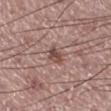Notes:
– follow-up — total-body-photography surveillance lesion; no biopsy
– automated metrics — an area of roughly 4 mm², an outline eccentricity of about 0.7 (0 = round, 1 = elongated), and two-axis asymmetry of about 0.2; a border-irregularity rating of about 2.5/10, internal color variation of about 4 on a 0–10 scale, and peripheral color asymmetry of about 1.5; a nevus-likeness score of about 45/100
– size — about 2.5 mm
– image source — ~15 mm tile from a whole-body skin photo
– illumination — white-light illumination
– site — the right lower leg
– patient — male, about 65 years old